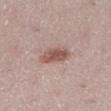  site: right lower leg
  patient:
    sex: female
    age_approx: 25
  lesion_size:
    long_diameter_mm_approx: 4.0
  image:
    source: total-body photography crop
    field_of_view_mm: 15
  lighting: white-light